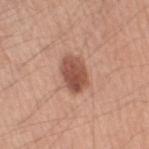Part of a total-body skin-imaging series; this lesion was reviewed on a skin check and was not flagged for biopsy.
The lesion is on the arm.
A close-up tile cropped from a whole-body skin photograph, about 15 mm across.
The patient is a male roughly 70 years of age.